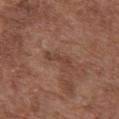* workup · catalogued during a skin exam; not biopsied
* anatomic site · the chest
* illumination · white-light
* image source · total-body-photography crop, ~15 mm field of view
* subject · female, aged around 75
* lesion size · about 3.5 mm